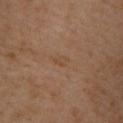location: the upper back | lighting: cross-polarized | image source: ~15 mm tile from a whole-body skin photo | lesion size: about 2 mm | TBP lesion metrics: a footprint of about 2.5 mm², a shape eccentricity near 0.8, and two-axis asymmetry of about 0.3; a mean CIELAB color near L≈46 a*≈17 b*≈31, about 4 CIELAB-L* units darker than the surrounding skin, and a normalized lesion–skin contrast near 4.5; a nevus-likeness score of about 0/100 and a lesion-detection confidence of about 100/100 | subject: male, aged 48 to 52.